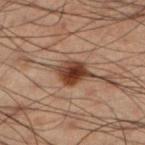Assessment:
Recorded during total-body skin imaging; not selected for excision or biopsy.
Background:
A male subject roughly 50 years of age. The lesion is located on the left lower leg. A region of skin cropped from a whole-body photographic capture, roughly 15 mm wide. Measured at roughly 4 mm in maximum diameter. The tile uses cross-polarized illumination.